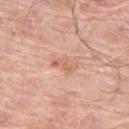biopsy status: no biopsy performed (imaged during a skin exam) | body site: the left thigh | imaging modality: 15 mm crop, total-body photography | illumination: white-light illumination | size: ~3 mm (longest diameter) | subject: male, in their 80s.